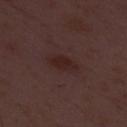A close-up tile cropped from a whole-body skin photograph, about 15 mm across.
A male subject, roughly 50 years of age.